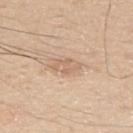location: the right upper arm
subject: male, approximately 30 years of age
image: ~15 mm tile from a whole-body skin photo
tile lighting: white-light illumination
lesion size: ~3 mm (longest diameter)